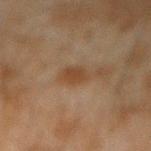Q: Lesion location?
A: the left forearm
Q: What did automated image analysis measure?
A: a nevus-likeness score of about 65/100 and lesion-presence confidence of about 100/100
Q: What is the imaging modality?
A: total-body-photography crop, ~15 mm field of view
Q: What lighting was used for the tile?
A: cross-polarized illumination
Q: Who is the patient?
A: male, aged around 45